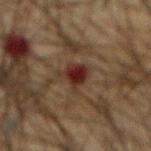Assessment:
Imaged during a routine full-body skin examination; the lesion was not biopsied and no histopathology is available.
Context:
The patient is a male about 65 years old. The lesion is located on the abdomen. A region of skin cropped from a whole-body photographic capture, roughly 15 mm wide. Automated image analysis of the tile measured a footprint of about 6 mm², an eccentricity of roughly 0.6, and a shape-asymmetry score of about 0.4 (0 = symmetric). The software also gave a lesion–skin lightness drop of about 9 and a normalized border contrast of about 11.